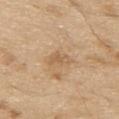Clinical impression: This lesion was catalogued during total-body skin photography and was not selected for biopsy. Image and clinical context: A 15 mm close-up extracted from a 3D total-body photography capture. Located on the upper back. Captured under white-light illumination. Longest diameter approximately 3 mm. A male subject, approximately 70 years of age.